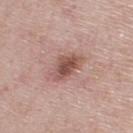Q: Was this lesion biopsied?
A: imaged on a skin check; not biopsied
Q: What kind of image is this?
A: 15 mm crop, total-body photography
Q: Lesion location?
A: the upper back
Q: Patient demographics?
A: male, in their mid-40s